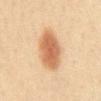biopsy status=total-body-photography surveillance lesion; no biopsy
subject=female, approximately 40 years of age
body site=the abdomen
image source=~15 mm crop, total-body skin-cancer survey